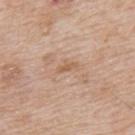{"biopsy_status": "not biopsied; imaged during a skin examination", "lesion_size": {"long_diameter_mm_approx": 2.5}, "site": "upper back", "patient": {"sex": "male", "age_approx": 65}, "image": {"source": "total-body photography crop", "field_of_view_mm": 15}, "lighting": "white-light"}